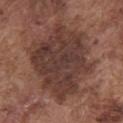Part of a total-body skin-imaging series; this lesion was reviewed on a skin check and was not flagged for biopsy.
The patient is a male aged around 75.
About 9 mm across.
From the chest.
A region of skin cropped from a whole-body photographic capture, roughly 15 mm wide.
Imaged with white-light lighting.